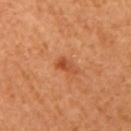biopsy status: imaged on a skin check; not biopsied | acquisition: ~15 mm crop, total-body skin-cancer survey | illumination: cross-polarized illumination | size: ~2.5 mm (longest diameter) | patient: female, aged around 40 | anatomic site: the right upper arm.